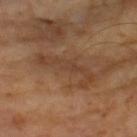Impression:
Captured during whole-body skin photography for melanoma surveillance; the lesion was not biopsied.
Background:
The subject is a male aged around 65. A 15 mm close-up extracted from a 3D total-body photography capture. Captured under cross-polarized illumination.